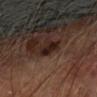Part of a total-body skin-imaging series; this lesion was reviewed on a skin check and was not flagged for biopsy. Automated tile analysis of the lesion measured a footprint of about 4 mm² and a shape eccentricity near 0.8. It also reported a mean CIELAB color near L≈20 a*≈16 b*≈19, about 10 CIELAB-L* units darker than the surrounding skin, and a normalized lesion–skin contrast near 11.5. And it measured a within-lesion color-variation index near 2/10 and radial color variation of about 1. The lesion is on the left forearm. Approximately 3 mm at its widest. The subject is a male aged 68–72. The tile uses cross-polarized illumination. A 15 mm close-up extracted from a 3D total-body photography capture.